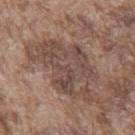biopsy_status: not biopsied; imaged during a skin examination
automated_metrics:
  area_mm2_approx: 27.0
  eccentricity: 0.85
  cielab_L: 46
  cielab_a: 16
  cielab_b: 23
  vs_skin_darker_L: 9.0
  vs_skin_contrast_norm: 7.0
  color_variation_0_10: 5.5
  peripheral_color_asymmetry: 2.0
image:
  source: total-body photography crop
  field_of_view_mm: 15
lighting: white-light
patient:
  sex: male
  age_approx: 75
site: mid back